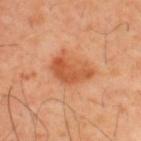follow-up: imaged on a skin check; not biopsied | site: the upper back | lesion size: about 4.5 mm | image: 15 mm crop, total-body photography | patient: male, approximately 40 years of age.